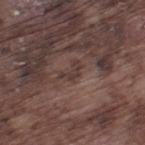  biopsy_status: not biopsied; imaged during a skin examination
  automated_metrics:
    cielab_L: 36
    cielab_a: 16
    cielab_b: 19
    vs_skin_darker_L: 6.0
    border_irregularity_0_10: 8.0
    color_variation_0_10: 0.0
    peripheral_color_asymmetry: 0.0
    nevus_likeness_0_100: 0
    lesion_detection_confidence_0_100: 70
  site: left thigh
  lesion_size:
    long_diameter_mm_approx: 2.5
  lighting: white-light
  patient:
    sex: male
    age_approx: 75
  image:
    source: total-body photography crop
    field_of_view_mm: 15